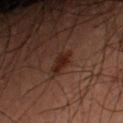The lesion was photographed on a routine skin check and not biopsied; there is no pathology result. The lesion is on the left upper arm. A male patient, roughly 60 years of age. A 15 mm close-up extracted from a 3D total-body photography capture.